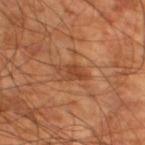A 15 mm close-up extracted from a 3D total-body photography capture. On the left thigh. The patient is a male about 60 years old.